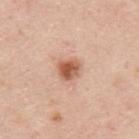Assessment: This lesion was catalogued during total-body skin photography and was not selected for biopsy. Background: Imaged with white-light lighting. Measured at roughly 2.5 mm in maximum diameter. A male subject, approximately 45 years of age. A roughly 15 mm field-of-view crop from a total-body skin photograph. From the back.